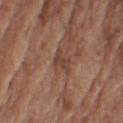- workup: no biopsy performed (imaged during a skin exam)
- site: the right upper arm
- diameter: about 2.5 mm
- subject: male, approximately 75 years of age
- illumination: white-light
- image: 15 mm crop, total-body photography